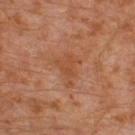anatomic site = the left leg; subject = male, aged approximately 30; imaging modality = ~15 mm crop, total-body skin-cancer survey; tile lighting = cross-polarized.